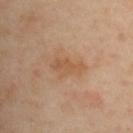Recorded during total-body skin imaging; not selected for excision or biopsy. The patient is a female aged approximately 40. A roughly 15 mm field-of-view crop from a total-body skin photograph. The lesion is on the upper back. The lesion-visualizer software estimated a mean CIELAB color near L≈55 a*≈20 b*≈35 and a normalized lesion–skin contrast near 6. And it measured an automated nevus-likeness rating near 0 out of 100 and a lesion-detection confidence of about 100/100.